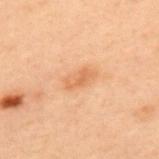Impression: Captured during whole-body skin photography for melanoma surveillance; the lesion was not biopsied. Acquisition and patient details: A 15 mm close-up extracted from a 3D total-body photography capture. The subject is a male aged approximately 55. About 3.5 mm across. Captured under cross-polarized illumination. The lesion is on the back.